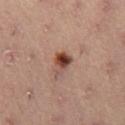Part of a total-body skin-imaging series; this lesion was reviewed on a skin check and was not flagged for biopsy.
This is a cross-polarized tile.
Located on the right thigh.
Cropped from a whole-body photographic skin survey; the tile spans about 15 mm.
A male subject aged 53 to 57.